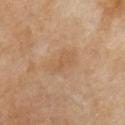<case>
<biopsy_status>not biopsied; imaged during a skin examination</biopsy_status>
<patient>
  <sex>female</sex>
  <age_approx>50</age_approx>
</patient>
<site>chest</site>
<image>
  <source>total-body photography crop</source>
  <field_of_view_mm>15</field_of_view_mm>
</image>
</case>